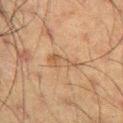Q: Is there a histopathology result?
A: imaged on a skin check; not biopsied
Q: Where on the body is the lesion?
A: the left thigh
Q: Who is the patient?
A: male, aged 58–62
Q: What did automated image analysis measure?
A: an area of roughly 5.5 mm², a shape eccentricity near 0.9, and a shape-asymmetry score of about 0.5 (0 = symmetric); a mean CIELAB color near L≈44 a*≈15 b*≈28, a lesion–skin lightness drop of about 6, and a normalized border contrast of about 5; border irregularity of about 5 on a 0–10 scale and internal color variation of about 3.5 on a 0–10 scale; a nevus-likeness score of about 0/100 and lesion-presence confidence of about 95/100
Q: What is the imaging modality?
A: total-body-photography crop, ~15 mm field of view